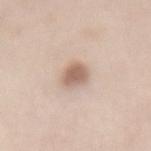No biopsy was performed on this lesion — it was imaged during a full skin examination and was not determined to be concerning. The subject is a female approximately 50 years of age. A 15 mm crop from a total-body photograph taken for skin-cancer surveillance. The lesion is on the mid back. Imaged with white-light lighting. Longest diameter approximately 3 mm. Automated image analysis of the tile measured a lesion color around L≈62 a*≈17 b*≈26 in CIELAB and a normalized border contrast of about 8. The software also gave border irregularity of about 1.5 on a 0–10 scale, internal color variation of about 2.5 on a 0–10 scale, and a peripheral color-asymmetry measure near 0.5. The software also gave a nevus-likeness score of about 90/100 and a detector confidence of about 100 out of 100 that the crop contains a lesion.